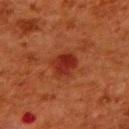workup: total-body-photography surveillance lesion; no biopsy | patient: female, aged 48–52 | body site: the upper back | imaging modality: total-body-photography crop, ~15 mm field of view | lighting: cross-polarized illumination | lesion size: ≈3 mm.